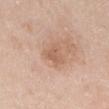| feature | finding |
|---|---|
| illumination | white-light |
| subject | male, approximately 65 years of age |
| site | the abdomen |
| TBP lesion metrics | a classifier nevus-likeness of about 0/100 |
| image source | total-body-photography crop, ~15 mm field of view |